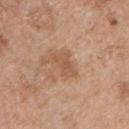No biopsy was performed on this lesion — it was imaged during a full skin examination and was not determined to be concerning.
The lesion-visualizer software estimated about 8 CIELAB-L* units darker than the surrounding skin and a lesion-to-skin contrast of about 6 (normalized; higher = more distinct).
A 15 mm close-up tile from a total-body photography series done for melanoma screening.
A male subject, in their mid- to late 50s.
From the front of the torso.
Captured under white-light illumination.
The recorded lesion diameter is about 3.5 mm.